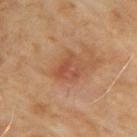Clinical impression:
Recorded during total-body skin imaging; not selected for excision or biopsy.
Context:
The subject is a male in their mid-60s. Measured at roughly 3.5 mm in maximum diameter. A 15 mm close-up extracted from a 3D total-body photography capture. The lesion is located on the arm. The tile uses cross-polarized illumination.